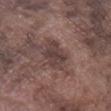| key | value |
|---|---|
| notes | catalogued during a skin exam; not biopsied |
| lighting | white-light |
| automated metrics | a footprint of about 9 mm² and a shape-asymmetry score of about 0.15 (0 = symmetric); roughly 9 lightness units darker than nearby skin and a normalized border contrast of about 8; a lesion-detection confidence of about 95/100 |
| body site | the right lower leg |
| subject | male, in their mid- to late 70s |
| diameter | about 4 mm |
| acquisition | ~15 mm tile from a whole-body skin photo |